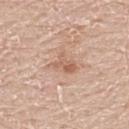biopsy status: catalogued during a skin exam; not biopsied
tile lighting: white-light
subject: male, in their 60s
imaging modality: 15 mm crop, total-body photography
diameter: about 3 mm
body site: the upper back